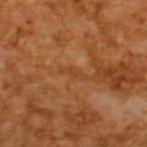follow-up = total-body-photography surveillance lesion; no biopsy | lesion diameter = ~3 mm (longest diameter) | tile lighting = cross-polarized illumination | image source = total-body-photography crop, ~15 mm field of view | TBP lesion metrics = an area of roughly 2 mm², a shape eccentricity near 0.95, and two-axis asymmetry of about 0.4; border irregularity of about 5 on a 0–10 scale and internal color variation of about 0 on a 0–10 scale | patient = male, approximately 65 years of age.